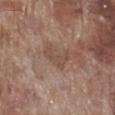Q: Is there a histopathology result?
A: total-body-photography surveillance lesion; no biopsy
Q: What kind of image is this?
A: 15 mm crop, total-body photography
Q: Lesion location?
A: the right lower leg
Q: Who is the patient?
A: male, approximately 70 years of age
Q: Automated lesion metrics?
A: a mean CIELAB color near L≈48 a*≈18 b*≈25, roughly 7 lightness units darker than nearby skin, and a normalized border contrast of about 5.5